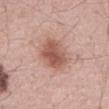Part of a total-body skin-imaging series; this lesion was reviewed on a skin check and was not flagged for biopsy. This is a white-light tile. The recorded lesion diameter is about 5 mm. A male subject, about 60 years old. On the abdomen. A close-up tile cropped from a whole-body skin photograph, about 15 mm across.